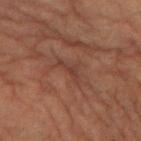notes: total-body-photography surveillance lesion; no biopsy | subject: female, aged approximately 80 | imaging modality: ~15 mm crop, total-body skin-cancer survey | lesion diameter: ≈3 mm | image-analysis metrics: a footprint of about 3 mm², an outline eccentricity of about 0.9 (0 = round, 1 = elongated), and a symmetry-axis asymmetry near 0.55; a mean CIELAB color near L≈28 a*≈17 b*≈20, a lesion–skin lightness drop of about 5, and a lesion-to-skin contrast of about 5 (normalized; higher = more distinct); a border-irregularity rating of about 6/10, a within-lesion color-variation index near 0/10, and a peripheral color-asymmetry measure near 0 | anatomic site: the leg.